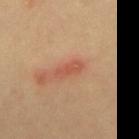Imaged during a routine full-body skin examination; the lesion was not biopsied and no histopathology is available. A 15 mm close-up tile from a total-body photography series done for melanoma screening. The total-body-photography lesion software estimated an area of roughly 4 mm², a shape eccentricity near 0.9, and two-axis asymmetry of about 0.3. It also reported a lesion color around L≈54 a*≈28 b*≈34 in CIELAB and a normalized border contrast of about 6. The software also gave an automated nevus-likeness rating near 0 out of 100 and a lesion-detection confidence of about 100/100. On the mid back. A male subject, aged 38–42.